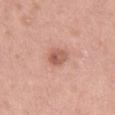{
  "biopsy_status": "not biopsied; imaged during a skin examination",
  "site": "right thigh",
  "image": {
    "source": "total-body photography crop",
    "field_of_view_mm": 15
  },
  "patient": {
    "sex": "female",
    "age_approx": 55
  },
  "lighting": "white-light",
  "lesion_size": {
    "long_diameter_mm_approx": 3.0
  }
}